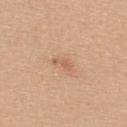workup: no biopsy performed (imaged during a skin exam) | subject: female, aged approximately 20 | tile lighting: white-light illumination | lesion size: ~2.5 mm (longest diameter) | acquisition: 15 mm crop, total-body photography | anatomic site: the upper back.